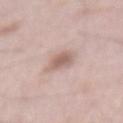Part of a total-body skin-imaging series; this lesion was reviewed on a skin check and was not flagged for biopsy.
The total-body-photography lesion software estimated a lesion color around L≈62 a*≈17 b*≈23 in CIELAB, roughly 11 lightness units darker than nearby skin, and a normalized lesion–skin contrast near 7.
About 4 mm across.
The lesion is on the mid back.
The patient is a male approximately 65 years of age.
The tile uses white-light illumination.
A close-up tile cropped from a whole-body skin photograph, about 15 mm across.